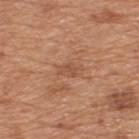biopsy status = no biopsy performed (imaged during a skin exam)
illumination = white-light
patient = male, aged approximately 65
diameter = about 2.5 mm
location = the upper back
image source = total-body-photography crop, ~15 mm field of view